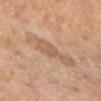The lesion was photographed on a routine skin check and not biopsied; there is no pathology result.
This is a cross-polarized tile.
Measured at roughly 6.5 mm in maximum diameter.
A female subject, aged around 70.
Located on the left lower leg.
This image is a 15 mm lesion crop taken from a total-body photograph.